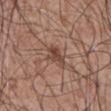Impression:
Recorded during total-body skin imaging; not selected for excision or biopsy.
Background:
This image is a 15 mm lesion crop taken from a total-body photograph. Captured under white-light illumination. A male subject aged around 60. Located on the abdomen. About 3 mm across. The lesion-visualizer software estimated a footprint of about 5 mm², an outline eccentricity of about 0.75 (0 = round, 1 = elongated), and a shape-asymmetry score of about 0.2 (0 = symmetric). The analysis additionally found an average lesion color of about L≈45 a*≈20 b*≈25 (CIELAB). And it measured a border-irregularity index near 2/10 and a within-lesion color-variation index near 4/10. And it measured a classifier nevus-likeness of about 5/100 and a detector confidence of about 100 out of 100 that the crop contains a lesion.